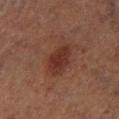patient:
  sex: male
  age_approx: 75
lighting: cross-polarized
lesion_size:
  long_diameter_mm_approx: 4.0
site: left lower leg
image:
  source: total-body photography crop
  field_of_view_mm: 15